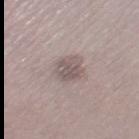Assessment:
Recorded during total-body skin imaging; not selected for excision or biopsy.
Context:
The total-body-photography lesion software estimated a lesion–skin lightness drop of about 9 and a lesion-to-skin contrast of about 6.5 (normalized; higher = more distinct). And it measured a lesion-detection confidence of about 60/100. Approximately 3.5 mm at its widest. Captured under white-light illumination. A female patient, aged around 40. A 15 mm close-up tile from a total-body photography series done for melanoma screening. The lesion is located on the leg.